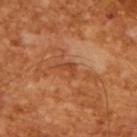biopsy_status: not biopsied; imaged during a skin examination
lesion_size:
  long_diameter_mm_approx: 2.5
patient:
  sex: male
  age_approx: 65
lighting: cross-polarized
image:
  source: total-body photography crop
  field_of_view_mm: 15
automated_metrics:
  cielab_L: 44
  cielab_a: 27
  cielab_b: 37
  vs_skin_darker_L: 7.0
  vs_skin_contrast_norm: 6.0
  border_irregularity_0_10: 5.0
  color_variation_0_10: 0.0
  nevus_likeness_0_100: 0
  lesion_detection_confidence_0_100: 100